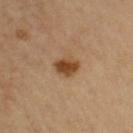| key | value |
|---|---|
| workup | total-body-photography surveillance lesion; no biopsy |
| acquisition | 15 mm crop, total-body photography |
| site | the right upper arm |
| lighting | cross-polarized illumination |
| size | about 3 mm |
| subject | female, in their mid-30s |